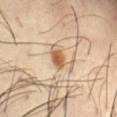Part of a total-body skin-imaging series; this lesion was reviewed on a skin check and was not flagged for biopsy.
Approximately 2.5 mm at its widest.
Cropped from a whole-body photographic skin survey; the tile spans about 15 mm.
The subject is a male roughly 40 years of age.
On the abdomen.